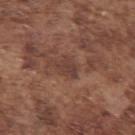workup: no biopsy performed (imaged during a skin exam)
subject: male, in their mid- to late 70s
anatomic site: the arm
imaging modality: total-body-photography crop, ~15 mm field of view
automated lesion analysis: a lesion area of about 4.5 mm² and an outline eccentricity of about 0.65 (0 = round, 1 = elongated); a mean CIELAB color near L≈39 a*≈19 b*≈23, roughly 7 lightness units darker than nearby skin, and a normalized border contrast of about 6.5; border irregularity of about 4 on a 0–10 scale, a within-lesion color-variation index near 1.5/10, and peripheral color asymmetry of about 0.5
tile lighting: white-light